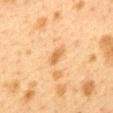follow-up: no biopsy performed (imaged during a skin exam) | acquisition: ~15 mm tile from a whole-body skin photo | patient: female, aged 38 to 42 | automated metrics: a lesion color around L≈55 a*≈19 b*≈39 in CIELAB, a lesion–skin lightness drop of about 9, and a lesion-to-skin contrast of about 7 (normalized; higher = more distinct) | body site: the back.